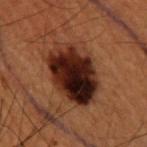Q: Was a biopsy performed?
A: catalogued during a skin exam; not biopsied
Q: What is the anatomic site?
A: the back
Q: Who is the patient?
A: male, aged 48–52
Q: How large is the lesion?
A: about 7 mm
Q: Automated lesion metrics?
A: an area of roughly 27 mm², a shape eccentricity near 0.7, and two-axis asymmetry of about 0.1; a border-irregularity rating of about 1.5/10, a color-variation rating of about 10/10, and peripheral color asymmetry of about 3.5
Q: How was this image acquired?
A: ~15 mm crop, total-body skin-cancer survey